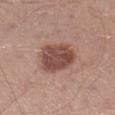Imaged during a routine full-body skin examination; the lesion was not biopsied and no histopathology is available. On the right lower leg. A region of skin cropped from a whole-body photographic capture, roughly 15 mm wide. The patient is a male approximately 30 years of age. Imaged with white-light lighting. Measured at roughly 5 mm in maximum diameter.